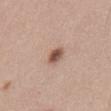The lesion was photographed on a routine skin check and not biopsied; there is no pathology result. Imaged with white-light lighting. A male subject, about 40 years old. The recorded lesion diameter is about 2.5 mm. A close-up tile cropped from a whole-body skin photograph, about 15 mm across. The lesion is located on the front of the torso.